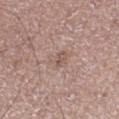Case summary:
- notes · imaged on a skin check; not biopsied
- lighting · white-light
- diameter · about 3 mm
- body site · the left lower leg
- imaging modality · total-body-photography crop, ~15 mm field of view
- subject · male, in their mid- to late 60s